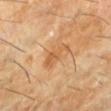<case>
<biopsy_status>not biopsied; imaged during a skin examination</biopsy_status>
<lesion_size>
  <long_diameter_mm_approx>3.5</long_diameter_mm_approx>
</lesion_size>
<site>left lower leg</site>
<lighting>cross-polarized</lighting>
<image>
  <source>total-body photography crop</source>
  <field_of_view_mm>15</field_of_view_mm>
</image>
<patient>
  <sex>male</sex>
  <age_approx>60</age_approx>
</patient>
</case>